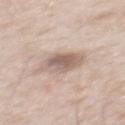The lesion was tiled from a total-body skin photograph and was not biopsied.
A 15 mm close-up extracted from a 3D total-body photography capture.
The lesion is on the mid back.
A male patient, aged 48 to 52.